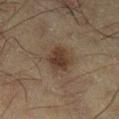notes: imaged on a skin check; not biopsied | acquisition: total-body-photography crop, ~15 mm field of view | location: the leg | illumination: cross-polarized | patient: male, aged 48 to 52 | image-analysis metrics: a footprint of about 8 mm²; a lesion-to-skin contrast of about 8.5 (normalized; higher = more distinct); a within-lesion color-variation index near 3/10 and radial color variation of about 1.